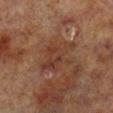Findings:
• workup: total-body-photography surveillance lesion; no biopsy
• lesion diameter: ~4 mm (longest diameter)
• tile lighting: cross-polarized illumination
• subject: male, aged 68 to 72
• body site: the right lower leg
• image source: 15 mm crop, total-body photography